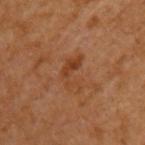Q: Was a biopsy performed?
A: imaged on a skin check; not biopsied
Q: What is the anatomic site?
A: the left upper arm
Q: Patient demographics?
A: male, aged approximately 65
Q: What kind of image is this?
A: ~15 mm tile from a whole-body skin photo